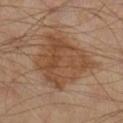notes — catalogued during a skin exam; not biopsied | acquisition — ~15 mm tile from a whole-body skin photo | lighting — cross-polarized illumination | site — the leg | automated metrics — a shape eccentricity near 0.45 and two-axis asymmetry of about 0.3 | subject — aged 53–57.